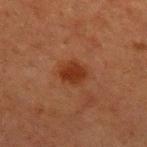| key | value |
|---|---|
| subject | male, about 65 years old |
| diameter | ~3.5 mm (longest diameter) |
| acquisition | ~15 mm tile from a whole-body skin photo |
| illumination | cross-polarized illumination |
| site | the right upper arm |